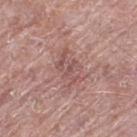Part of a total-body skin-imaging series; this lesion was reviewed on a skin check and was not flagged for biopsy. This image is a 15 mm lesion crop taken from a total-body photograph. This is a white-light tile. The lesion is on the left thigh. A male patient, aged around 65.